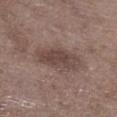Impression: This lesion was catalogued during total-body skin photography and was not selected for biopsy. Acquisition and patient details: The recorded lesion diameter is about 5.5 mm. Automated tile analysis of the lesion measured an area of roughly 13 mm², an eccentricity of roughly 0.9, and two-axis asymmetry of about 0.15. And it measured an automated nevus-likeness rating near 20 out of 100. Cropped from a whole-body photographic skin survey; the tile spans about 15 mm. The lesion is located on the right lower leg. A male patient, about 70 years old. This is a white-light tile.